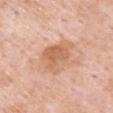biopsy_status: not biopsied; imaged during a skin examination
lesion_size:
  long_diameter_mm_approx: 4.5
image:
  source: total-body photography crop
  field_of_view_mm: 15
patient:
  sex: male
  age_approx: 55
lighting: white-light
site: arm